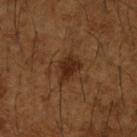Cropped from a whole-body photographic skin survey; the tile spans about 15 mm. Captured under cross-polarized illumination. A male patient approximately 65 years of age. From the right forearm. Longest diameter approximately 3.5 mm.